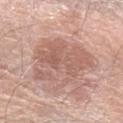Longest diameter approximately 7.5 mm. The tile uses white-light illumination. A male patient, roughly 70 years of age. A region of skin cropped from a whole-body photographic capture, roughly 15 mm wide. Automated image analysis of the tile measured an eccentricity of roughly 0.65 and two-axis asymmetry of about 0.35. The analysis additionally found a mean CIELAB color near L≈59 a*≈21 b*≈26, a lesion–skin lightness drop of about 9, and a normalized border contrast of about 6. It also reported internal color variation of about 4 on a 0–10 scale and peripheral color asymmetry of about 1.5. The lesion is located on the left forearm.